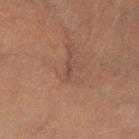Notes:
– biopsy status · imaged on a skin check; not biopsied
– anatomic site · the left lower leg
– illumination · cross-polarized
– imaging modality · ~15 mm crop, total-body skin-cancer survey
– patient · male, approximately 60 years of age